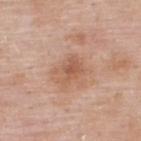notes — catalogued during a skin exam; not biopsied | patient — male, roughly 55 years of age | anatomic site — the back | illumination — white-light illumination | image source — 15 mm crop, total-body photography.